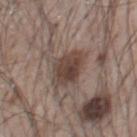Acquisition and patient details:
Captured under white-light illumination. This image is a 15 mm lesion crop taken from a total-body photograph. A male patient aged around 55. The lesion's longest dimension is about 4 mm. Located on the chest.